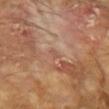This lesion was catalogued during total-body skin photography and was not selected for biopsy. On the left forearm. Automated image analysis of the tile measured border irregularity of about 4 on a 0–10 scale and radial color variation of about 0. This image is a 15 mm lesion crop taken from a total-body photograph. Captured under cross-polarized illumination. The subject is a male aged 68 to 72.